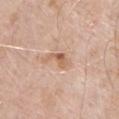Notes:
* subject · male, roughly 70 years of age
* imaging modality · ~15 mm crop, total-body skin-cancer survey
* site · the head or neck